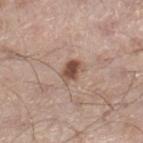Background: A lesion tile, about 15 mm wide, cut from a 3D total-body photograph. Captured under white-light illumination. Measured at roughly 3 mm in maximum diameter. Automated tile analysis of the lesion measured a lesion area of about 5 mm², a shape eccentricity near 0.7, and two-axis asymmetry of about 0.25. The software also gave a nevus-likeness score of about 95/100 and a lesion-detection confidence of about 100/100. The patient is a male in their mid- to late 30s. The lesion is on the right lower leg.